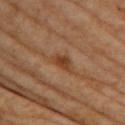Q: What is the anatomic site?
A: the upper back
Q: How was the tile lit?
A: cross-polarized illumination
Q: Who is the patient?
A: female, approximately 65 years of age
Q: What kind of image is this?
A: 15 mm crop, total-body photography
Q: How large is the lesion?
A: ≈3 mm